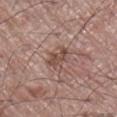No biopsy was performed on this lesion — it was imaged during a full skin examination and was not determined to be concerning. The lesion is on the right lower leg. A male subject aged approximately 70. This is a white-light tile. The lesion-visualizer software estimated a lesion area of about 6 mm², an eccentricity of roughly 0.65, and two-axis asymmetry of about 0.35. And it measured a mean CIELAB color near L≈48 a*≈18 b*≈23 and a normalized border contrast of about 7. And it measured a classifier nevus-likeness of about 5/100 and a detector confidence of about 100 out of 100 that the crop contains a lesion. A close-up tile cropped from a whole-body skin photograph, about 15 mm across.